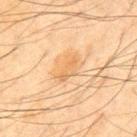| field | value |
|---|---|
| follow-up | no biopsy performed (imaged during a skin exam) |
| site | the mid back |
| patient | male, aged 43 to 47 |
| diameter | ≈3.5 mm |
| image | ~15 mm tile from a whole-body skin photo |
| automated lesion analysis | about 6 CIELAB-L* units darker than the surrounding skin and a lesion-to-skin contrast of about 5.5 (normalized; higher = more distinct) |